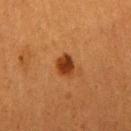Q: Is there a histopathology result?
A: no biopsy performed (imaged during a skin exam)
Q: How was this image acquired?
A: ~15 mm crop, total-body skin-cancer survey
Q: Who is the patient?
A: female, roughly 55 years of age
Q: How large is the lesion?
A: ~2.5 mm (longest diameter)
Q: How was the tile lit?
A: cross-polarized
Q: Lesion location?
A: the right upper arm